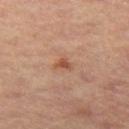Assessment: The lesion was photographed on a routine skin check and not biopsied; there is no pathology result. Image and clinical context: Imaged with cross-polarized lighting. A male subject aged around 60. Measured at roughly 2 mm in maximum diameter. Located on the leg. A 15 mm close-up tile from a total-body photography series done for melanoma screening.